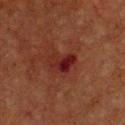Notes:
* notes — total-body-photography surveillance lesion; no biopsy
* patient — male, aged around 75
* image — ~15 mm tile from a whole-body skin photo
* location — the chest